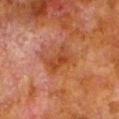Q: Is there a histopathology result?
A: imaged on a skin check; not biopsied
Q: What did automated image analysis measure?
A: an area of roughly 7 mm² and an outline eccentricity of about 0.8 (0 = round, 1 = elongated); border irregularity of about 3.5 on a 0–10 scale, internal color variation of about 3.5 on a 0–10 scale, and radial color variation of about 1
Q: What is the lesion's diameter?
A: about 3.5 mm
Q: What is the anatomic site?
A: the left lower leg
Q: What kind of image is this?
A: 15 mm crop, total-body photography
Q: Who is the patient?
A: male, about 80 years old
Q: Illumination type?
A: cross-polarized